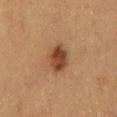No biopsy was performed on this lesion — it was imaged during a full skin examination and was not determined to be concerning.
The tile uses cross-polarized illumination.
The lesion's longest dimension is about 4 mm.
The total-body-photography lesion software estimated an eccentricity of roughly 0.75 and two-axis asymmetry of about 0.15. The software also gave an average lesion color of about L≈36 a*≈18 b*≈28 (CIELAB) and about 11 CIELAB-L* units darker than the surrounding skin. The software also gave a border-irregularity rating of about 1.5/10 and radial color variation of about 1. The analysis additionally found a lesion-detection confidence of about 100/100.
This image is a 15 mm lesion crop taken from a total-body photograph.
From the mid back.
A female subject, aged 38 to 42.